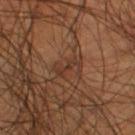The lesion is located on the leg. A male subject aged approximately 55. A close-up tile cropped from a whole-body skin photograph, about 15 mm across. Measured at roughly 3 mm in maximum diameter. Imaged with cross-polarized lighting.